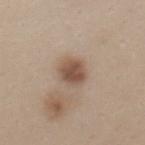The lesion was tiled from a total-body skin photograph and was not biopsied. A female patient aged 43–47. From the chest. The tile uses white-light illumination. A close-up tile cropped from a whole-body skin photograph, about 15 mm across.